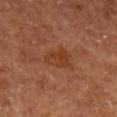| field | value |
|---|---|
| subject | male, aged around 65 |
| automated lesion analysis | a footprint of about 7 mm² and an outline eccentricity of about 0.7 (0 = round, 1 = elongated); border irregularity of about 3.5 on a 0–10 scale, a within-lesion color-variation index near 2.5/10, and radial color variation of about 1 |
| image source | 15 mm crop, total-body photography |
| diameter | ≈3.5 mm |
| body site | the leg |